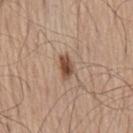notes = total-body-photography surveillance lesion; no biopsy
imaging modality = 15 mm crop, total-body photography
subject = male, aged approximately 70
automated metrics = a lesion area of about 4 mm², an eccentricity of roughly 0.8, and a symmetry-axis asymmetry near 0.25
diameter = ~3 mm (longest diameter)
tile lighting = white-light
anatomic site = the mid back